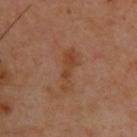follow-up = no biopsy performed (imaged during a skin exam) | site = the back | illumination = cross-polarized | lesion size = about 5 mm | subject = male, aged around 40 | image source = total-body-photography crop, ~15 mm field of view.